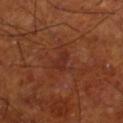Notes:
- notes: imaged on a skin check; not biopsied
- image: total-body-photography crop, ~15 mm field of view
- patient: male, roughly 70 years of age
- location: the right thigh